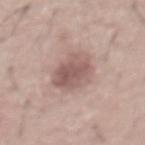{
  "biopsy_status": "not biopsied; imaged during a skin examination",
  "image": {
    "source": "total-body photography crop",
    "field_of_view_mm": 15
  },
  "site": "abdomen",
  "lesion_size": {
    "long_diameter_mm_approx": 5.0
  },
  "patient": {
    "sex": "male",
    "age_approx": 45
  },
  "lighting": "white-light"
}